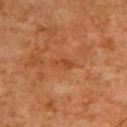Q: Was a biopsy performed?
A: no biopsy performed (imaged during a skin exam)
Q: What kind of image is this?
A: 15 mm crop, total-body photography
Q: Where on the body is the lesion?
A: the upper back
Q: What are the patient's age and sex?
A: male, aged around 65
Q: How was the tile lit?
A: cross-polarized illumination
Q: What is the lesion's diameter?
A: ≈2.5 mm